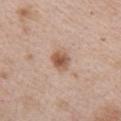Assessment:
The lesion was photographed on a routine skin check and not biopsied; there is no pathology result.
Context:
This is a white-light tile. A male subject aged around 65. A close-up tile cropped from a whole-body skin photograph, about 15 mm across. The lesion is on the chest.